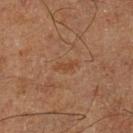Case summary:
• workup · catalogued during a skin exam; not biopsied
• patient · male, in their mid- to late 60s
• image · total-body-photography crop, ~15 mm field of view
• lesion diameter · about 2.5 mm
• site · the leg
• illumination · cross-polarized illumination